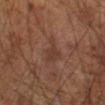Impression: No biopsy was performed on this lesion — it was imaged during a full skin examination and was not determined to be concerning. Context: A male subject, aged 63–67. A 15 mm crop from a total-body photograph taken for skin-cancer surveillance. Approximately 3 mm at its widest. Located on the left arm.